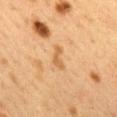Cropped from a whole-body photographic skin survey; the tile spans about 15 mm.
About 3 mm across.
A female subject in their 40s.
The total-body-photography lesion software estimated a lesion area of about 3 mm², an eccentricity of roughly 0.9, and a shape-asymmetry score of about 0.55 (0 = symmetric). The software also gave an average lesion color of about L≈51 a*≈19 b*≈37 (CIELAB), roughly 8 lightness units darker than nearby skin, and a lesion-to-skin contrast of about 6.5 (normalized; higher = more distinct).
Located on the mid back.
This is a cross-polarized tile.